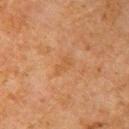patient: male, approximately 65 years of age
body site: the right upper arm
acquisition: total-body-photography crop, ~15 mm field of view